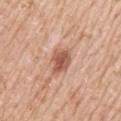Q: Was a biopsy performed?
A: catalogued during a skin exam; not biopsied
Q: How large is the lesion?
A: ≈3.5 mm
Q: What did automated image analysis measure?
A: border irregularity of about 3 on a 0–10 scale and a peripheral color-asymmetry measure near 1
Q: Who is the patient?
A: male, approximately 65 years of age
Q: What kind of image is this?
A: ~15 mm tile from a whole-body skin photo
Q: Where on the body is the lesion?
A: the left upper arm